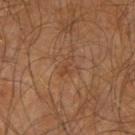This lesion was catalogued during total-body skin photography and was not selected for biopsy. This image is a 15 mm lesion crop taken from a total-body photograph. The tile uses cross-polarized illumination. About 2.5 mm across. A male subject aged 63–67. The lesion is on the right upper arm. Automated tile analysis of the lesion measured an average lesion color of about L≈40 a*≈20 b*≈31 (CIELAB), a lesion–skin lightness drop of about 5, and a normalized lesion–skin contrast near 5. And it measured border irregularity of about 5.5 on a 0–10 scale, internal color variation of about 0 on a 0–10 scale, and peripheral color asymmetry of about 0.